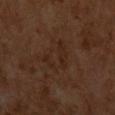anatomic site: the chest | illumination: cross-polarized | image: ~15 mm crop, total-body skin-cancer survey | patient: male, aged around 60 | image-analysis metrics: an area of roughly 7.5 mm² and an eccentricity of roughly 0.75; about 4 CIELAB-L* units darker than the surrounding skin and a normalized lesion–skin contrast near 4.5; a peripheral color-asymmetry measure near 0.5 | size: about 4.5 mm.